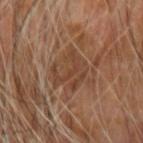biopsy status: imaged on a skin check; not biopsied
subject: male, in their mid-60s
tile lighting: cross-polarized
acquisition: 15 mm crop, total-body photography
site: the arm
size: ≈4.5 mm
TBP lesion metrics: an eccentricity of roughly 0.7; roughly 7 lightness units darker than nearby skin; a within-lesion color-variation index near 2/10 and a peripheral color-asymmetry measure near 0.5; a classifier nevus-likeness of about 0/100 and a lesion-detection confidence of about 70/100